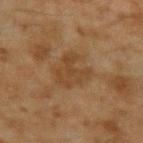Notes:
• follow-up — catalogued during a skin exam; not biopsied
• image — ~15 mm crop, total-body skin-cancer survey
• anatomic site — the arm
• lighting — cross-polarized
• subject — male, in their mid-40s
• automated lesion analysis — a footprint of about 12 mm² and a symmetry-axis asymmetry near 0.35
• lesion diameter — about 5 mm